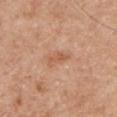No biopsy was performed on this lesion — it was imaged during a full skin examination and was not determined to be concerning.
The subject is a male roughly 60 years of age.
The lesion is located on the back.
Automated tile analysis of the lesion measured a border-irregularity rating of about 2.5/10 and a peripheral color-asymmetry measure near 0.5. It also reported an automated nevus-likeness rating near 0 out of 100.
About 3 mm across.
Imaged with white-light lighting.
A region of skin cropped from a whole-body photographic capture, roughly 15 mm wide.